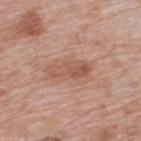The lesion was photographed on a routine skin check and not biopsied; there is no pathology result. Located on the upper back. Cropped from a total-body skin-imaging series; the visible field is about 15 mm. A male subject, about 60 years old. The tile uses white-light illumination. Longest diameter approximately 5 mm.